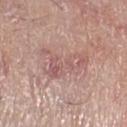biopsy status — imaged on a skin check; not biopsied
image — 15 mm crop, total-body photography
automated metrics — a footprint of about 14 mm², a shape eccentricity near 0.85, and a symmetry-axis asymmetry near 0.5; a border-irregularity rating of about 6.5/10, internal color variation of about 5 on a 0–10 scale, and a peripheral color-asymmetry measure near 1.5; a nevus-likeness score of about 0/100 and lesion-presence confidence of about 95/100
body site — the right lower leg
patient — male, about 55 years old
illumination — white-light illumination